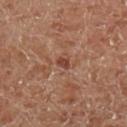follow-up: catalogued during a skin exam; not biopsied
anatomic site: the leg
image: total-body-photography crop, ~15 mm field of view
size: ≈2 mm
illumination: cross-polarized illumination
subject: male, in their mid- to late 50s